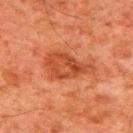Clinical impression: Captured during whole-body skin photography for melanoma surveillance; the lesion was not biopsied. Background: The lesion is located on the upper back. A male subject, aged 78–82. Cropped from a whole-body photographic skin survey; the tile spans about 15 mm.